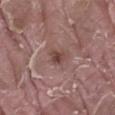notes: imaged on a skin check; not biopsied
imaging modality: ~15 mm tile from a whole-body skin photo
anatomic site: the right thigh
subject: male, aged around 40
tile lighting: white-light
TBP lesion metrics: an outline eccentricity of about 0.75 (0 = round, 1 = elongated) and a shape-asymmetry score of about 0.25 (0 = symmetric); border irregularity of about 2.5 on a 0–10 scale, internal color variation of about 2 on a 0–10 scale, and radial color variation of about 1
size: about 2.5 mm